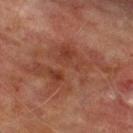No biopsy was performed on this lesion — it was imaged during a full skin examination and was not determined to be concerning.
A close-up tile cropped from a whole-body skin photograph, about 15 mm across.
A male patient about 75 years old.
From the leg.